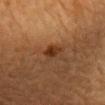Recorded during total-body skin imaging; not selected for excision or biopsy.
Automated tile analysis of the lesion measured border irregularity of about 3 on a 0–10 scale and radial color variation of about 1. The software also gave an automated nevus-likeness rating near 95 out of 100 and lesion-presence confidence of about 100/100.
A 15 mm close-up tile from a total-body photography series done for melanoma screening.
The lesion's longest dimension is about 2.5 mm.
A male patient aged 83 or over.
The lesion is on the chest.